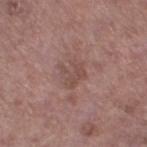{"biopsy_status": "not biopsied; imaged during a skin examination", "lighting": "white-light", "site": "left thigh", "lesion_size": {"long_diameter_mm_approx": 3.0}, "patient": {"sex": "male", "age_approx": 70}, "image": {"source": "total-body photography crop", "field_of_view_mm": 15}}